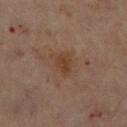<lesion>
<biopsy_status>not biopsied; imaged during a skin examination</biopsy_status>
<patient>
  <sex>female</sex>
  <age_approx>60</age_approx>
</patient>
<automated_metrics>
  <cielab_L>40</cielab_L>
  <cielab_a>17</cielab_a>
  <cielab_b>29</cielab_b>
  <vs_skin_contrast_norm>6.5</vs_skin_contrast_norm>
  <nevus_likeness_0_100>0</nevus_likeness_0_100>
  <lesion_detection_confidence_0_100>100</lesion_detection_confidence_0_100>
</automated_metrics>
<lighting>cross-polarized</lighting>
<site>right lower leg</site>
<image>
  <source>total-body photography crop</source>
  <field_of_view_mm>15</field_of_view_mm>
</image>
<lesion_size>
  <long_diameter_mm_approx>3.0</long_diameter_mm_approx>
</lesion_size>
</lesion>